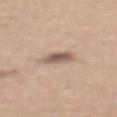Assessment: This lesion was catalogued during total-body skin photography and was not selected for biopsy. Clinical summary: A female subject, aged 53–57. The lesion is on the upper back. A roughly 15 mm field-of-view crop from a total-body skin photograph. The lesion-visualizer software estimated a lesion area of about 5 mm², a shape eccentricity near 0.7, and a symmetry-axis asymmetry near 0.2. The analysis additionally found a border-irregularity rating of about 2/10, a color-variation rating of about 4/10, and a peripheral color-asymmetry measure near 1.5.